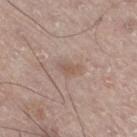- notes · no biopsy performed (imaged during a skin exam)
- lighting · white-light
- anatomic site · the right leg
- subject · male, aged approximately 70
- lesion size · ~3 mm (longest diameter)
- acquisition · total-body-photography crop, ~15 mm field of view